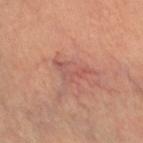notes=no biopsy performed (imaged during a skin exam) | subject=female, aged 43 to 47 | TBP lesion metrics=border irregularity of about 8.5 on a 0–10 scale, a color-variation rating of about 1.5/10, and radial color variation of about 0.5 | lesion diameter=≈4.5 mm | image=total-body-photography crop, ~15 mm field of view | illumination=cross-polarized illumination | location=the right leg.